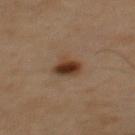workup: catalogued during a skin exam; not biopsied | patient: male, aged around 45 | acquisition: ~15 mm crop, total-body skin-cancer survey | location: the upper back | lesion diameter: ≈3 mm | illumination: cross-polarized illumination.